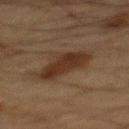biopsy status=no biopsy performed (imaged during a skin exam)
patient=male, aged approximately 65
lighting=cross-polarized
automated metrics=an average lesion color of about L≈26 a*≈14 b*≈23 (CIELAB) and a lesion–skin lightness drop of about 8; a color-variation rating of about 3/10
body site=the mid back
image source=total-body-photography crop, ~15 mm field of view
size=≈6 mm